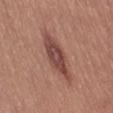The lesion was photographed on a routine skin check and not biopsied; there is no pathology result. The lesion-visualizer software estimated a footprint of about 6.5 mm² and a symmetry-axis asymmetry near 0.25. And it measured border irregularity of about 3.5 on a 0–10 scale and a color-variation rating of about 3/10. And it measured a nevus-likeness score of about 70/100 and a detector confidence of about 65 out of 100 that the crop contains a lesion. The patient is a female aged approximately 55. A roughly 15 mm field-of-view crop from a total-body skin photograph. The lesion is on the left thigh. About 5 mm across.